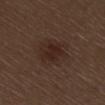This lesion was catalogued during total-body skin photography and was not selected for biopsy. Imaged with white-light lighting. Longest diameter approximately 3.5 mm. A male patient, roughly 70 years of age. Automated image analysis of the tile measured a lesion area of about 9 mm² and an eccentricity of roughly 0.35. The software also gave a mean CIELAB color near L≈24 a*≈16 b*≈21, about 6 CIELAB-L* units darker than the surrounding skin, and a lesion-to-skin contrast of about 7 (normalized; higher = more distinct). It also reported a border-irregularity rating of about 2.5/10 and radial color variation of about 1. The lesion is on the right thigh. Cropped from a whole-body photographic skin survey; the tile spans about 15 mm.